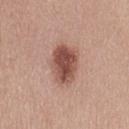Findings:
• notes · imaged on a skin check; not biopsied
• image source · 15 mm crop, total-body photography
• site · the lower back
• patient · female, about 55 years old
• diameter · about 5 mm
• image-analysis metrics · a footprint of about 13 mm² and an eccentricity of roughly 0.7
• tile lighting · white-light illumination